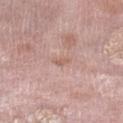notes: total-body-photography surveillance lesion; no biopsy
lighting: white-light
diameter: ≈2.5 mm
image source: ~15 mm tile from a whole-body skin photo
anatomic site: the left lower leg
patient: female, about 70 years old